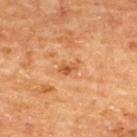Imaged during a routine full-body skin examination; the lesion was not biopsied and no histopathology is available.
Imaged with cross-polarized lighting.
Located on the upper back.
The patient is a male in their mid- to late 60s.
Cropped from a whole-body photographic skin survey; the tile spans about 15 mm.
Longest diameter approximately 2.5 mm.